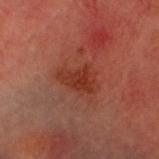Notes:
- workup: total-body-photography surveillance lesion; no biopsy
- imaging modality: ~15 mm tile from a whole-body skin photo
- subject: male, in their 60s
- body site: the head or neck
- tile lighting: cross-polarized illumination
- diameter: about 4 mm